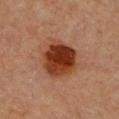Notes:
- follow-up: imaged on a skin check; not biopsied
- location: the chest
- patient: female, roughly 45 years of age
- image source: ~15 mm crop, total-body skin-cancer survey
- illumination: cross-polarized
- automated metrics: an average lesion color of about L≈32 a*≈24 b*≈30 (CIELAB) and about 14 CIELAB-L* units darker than the surrounding skin; a border-irregularity rating of about 1.5/10 and a peripheral color-asymmetry measure near 2; a classifier nevus-likeness of about 100/100